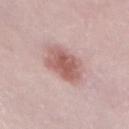biopsy_status: not biopsied; imaged during a skin examination
automated_metrics:
  cielab_L: 59
  cielab_a: 22
  cielab_b: 23
  vs_skin_darker_L: 12.0
  vs_skin_contrast_norm: 8.0
  nevus_likeness_0_100: 80
  lesion_detection_confidence_0_100: 100
patient:
  sex: female
  age_approx: 65
site: front of the torso
lesion_size:
  long_diameter_mm_approx: 4.0
lighting: white-light
image:
  source: total-body photography crop
  field_of_view_mm: 15